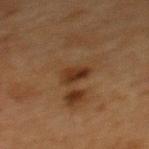Clinical impression: No biopsy was performed on this lesion — it was imaged during a full skin examination and was not determined to be concerning. Clinical summary: A roughly 15 mm field-of-view crop from a total-body skin photograph. The lesion is on the upper back. This is a cross-polarized tile. A male patient about 65 years old. About 2.5 mm across.